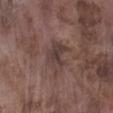Part of a total-body skin-imaging series; this lesion was reviewed on a skin check and was not flagged for biopsy. A male patient, aged 73 to 77. The lesion's longest dimension is about 4 mm. The lesion is on the left lower leg. This is a white-light tile. A lesion tile, about 15 mm wide, cut from a 3D total-body photograph.